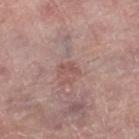The lesion was photographed on a routine skin check and not biopsied; there is no pathology result.
On the leg.
A roughly 15 mm field-of-view crop from a total-body skin photograph.
A female patient aged around 60.
The lesion's longest dimension is about 2.5 mm.
Imaged with white-light lighting.